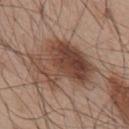<case>
  <patient>
    <sex>male</sex>
    <age_approx>55</age_approx>
  </patient>
  <lighting>white-light</lighting>
  <automated_metrics>
    <cielab_L>44</cielab_L>
    <cielab_a>19</cielab_a>
    <cielab_b>26</cielab_b>
    <vs_skin_darker_L>13.0</vs_skin_darker_L>
    <vs_skin_contrast_norm>10.0</vs_skin_contrast_norm>
    <border_irregularity_0_10>5.0</border_irregularity_0_10>
    <color_variation_0_10>8.5</color_variation_0_10>
    <peripheral_color_asymmetry>3.5</peripheral_color_asymmetry>
  </automated_metrics>
  <site>upper back</site>
  <image>
    <source>total-body photography crop</source>
    <field_of_view_mm>15</field_of_view_mm>
  </image>
  <lesion_size>
    <long_diameter_mm_approx>7.5</long_diameter_mm_approx>
  </lesion_size>
</case>